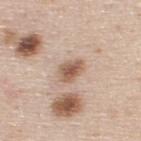Notes:
* workup: imaged on a skin check; not biopsied
* anatomic site: the upper back
* subject: male, aged 43 to 47
* imaging modality: ~15 mm tile from a whole-body skin photo
* lesion diameter: about 3.5 mm
* lighting: white-light illumination